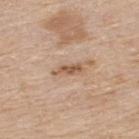Clinical impression:
Recorded during total-body skin imaging; not selected for excision or biopsy.
Image and clinical context:
The tile uses white-light illumination. An algorithmic analysis of the crop reported a lesion area of about 4 mm². The analysis additionally found a border-irregularity index near 4/10, a within-lesion color-variation index near 2/10, and a peripheral color-asymmetry measure near 0.5. A male subject, aged 78–82. The lesion's longest dimension is about 3.5 mm. Cropped from a total-body skin-imaging series; the visible field is about 15 mm. The lesion is on the upper back.